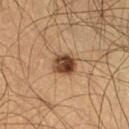Impression: Part of a total-body skin-imaging series; this lesion was reviewed on a skin check and was not flagged for biopsy. Context: Imaged with cross-polarized lighting. Automated image analysis of the tile measured a shape eccentricity near 0.45 and a symmetry-axis asymmetry near 0.2. The analysis additionally found a mean CIELAB color near L≈36 a*≈16 b*≈27. A male subject aged 63 to 67. A close-up tile cropped from a whole-body skin photograph, about 15 mm across. Approximately 3 mm at its widest. Located on the leg.